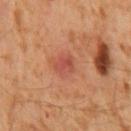<record>
<biopsy_status>not biopsied; imaged during a skin examination</biopsy_status>
<automated_metrics>
  <area_mm2_approx>4.0</area_mm2_approx>
  <eccentricity>0.75</eccentricity>
  <shape_asymmetry>0.3</shape_asymmetry>
  <cielab_L>45</cielab_L>
  <cielab_a>28</cielab_a>
  <cielab_b>29</cielab_b>
  <vs_skin_darker_L>8.0</vs_skin_darker_L>
  <vs_skin_contrast_norm>6.0</vs_skin_contrast_norm>
</automated_metrics>
<site>mid back</site>
<patient>
  <sex>male</sex>
  <age_approx>60</age_approx>
</patient>
<image>
  <source>total-body photography crop</source>
  <field_of_view_mm>15</field_of_view_mm>
</image>
<lesion_size>
  <long_diameter_mm_approx>3.0</long_diameter_mm_approx>
</lesion_size>
<lighting>cross-polarized</lighting>
</record>